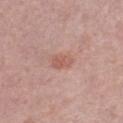Assessment: Part of a total-body skin-imaging series; this lesion was reviewed on a skin check and was not flagged for biopsy. Clinical summary: A region of skin cropped from a whole-body photographic capture, roughly 15 mm wide. Approximately 2.5 mm at its widest. Imaged with white-light lighting. Automated image analysis of the tile measured a footprint of about 3.5 mm², an outline eccentricity of about 0.8 (0 = round, 1 = elongated), and a symmetry-axis asymmetry near 0.3. The software also gave an average lesion color of about L≈57 a*≈23 b*≈26 (CIELAB) and roughly 8 lightness units darker than nearby skin. The analysis additionally found border irregularity of about 3 on a 0–10 scale and a within-lesion color-variation index near 1.5/10. And it measured a classifier nevus-likeness of about 10/100 and lesion-presence confidence of about 100/100. A female patient, roughly 50 years of age. On the right lower leg.